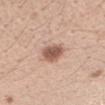Q: Was a biopsy performed?
A: imaged on a skin check; not biopsied
Q: Patient demographics?
A: female, aged 43–47
Q: Illumination type?
A: white-light
Q: What is the imaging modality?
A: total-body-photography crop, ~15 mm field of view
Q: Lesion location?
A: the left forearm
Q: Automated lesion metrics?
A: a lesion–skin lightness drop of about 15 and a lesion-to-skin contrast of about 9.5 (normalized; higher = more distinct); border irregularity of about 1.5 on a 0–10 scale and a within-lesion color-variation index near 3/10; an automated nevus-likeness rating near 80 out of 100 and lesion-presence confidence of about 100/100